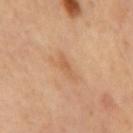The lesion was photographed on a routine skin check and not biopsied; there is no pathology result.
Imaged with cross-polarized lighting.
The lesion's longest dimension is about 3.5 mm.
An algorithmic analysis of the crop reported an area of roughly 3 mm² and a symmetry-axis asymmetry near 0.45. The software also gave a classifier nevus-likeness of about 0/100 and a detector confidence of about 100 out of 100 that the crop contains a lesion.
Cropped from a total-body skin-imaging series; the visible field is about 15 mm.
The lesion is located on the back.